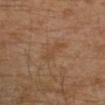Q: Was a biopsy performed?
A: imaged on a skin check; not biopsied
Q: Lesion location?
A: the left leg
Q: Automated lesion metrics?
A: a lesion color around L≈44 a*≈17 b*≈32 in CIELAB, roughly 5 lightness units darker than nearby skin, and a normalized lesion–skin contrast near 5; border irregularity of about 4.5 on a 0–10 scale and radial color variation of about 0; a lesion-detection confidence of about 100/100
Q: Patient demographics?
A: male, aged 28–32
Q: How was the tile lit?
A: cross-polarized
Q: How was this image acquired?
A: 15 mm crop, total-body photography
Q: Lesion size?
A: about 3.5 mm